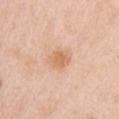follow-up: total-body-photography surveillance lesion; no biopsy | anatomic site: the arm | patient: female, in their 50s | image source: ~15 mm tile from a whole-body skin photo.